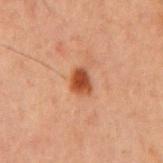Impression:
No biopsy was performed on this lesion — it was imaged during a full skin examination and was not determined to be concerning.
Acquisition and patient details:
A male subject, aged 58–62. The lesion-visualizer software estimated an outline eccentricity of about 0.65 (0 = round, 1 = elongated) and two-axis asymmetry of about 0.25. The software also gave a nevus-likeness score of about 100/100 and a lesion-detection confidence of about 100/100. The lesion is located on the mid back. A close-up tile cropped from a whole-body skin photograph, about 15 mm across.